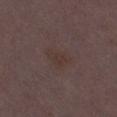| key | value |
|---|---|
| workup | no biopsy performed (imaged during a skin exam) |
| image | total-body-photography crop, ~15 mm field of view |
| patient | female, about 30 years old |
| lesion size | about 3.5 mm |
| site | the right thigh |